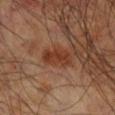Q: Was a biopsy performed?
A: no biopsy performed (imaged during a skin exam)
Q: Who is the patient?
A: male, in their 60s
Q: What is the anatomic site?
A: the right leg
Q: What is the imaging modality?
A: 15 mm crop, total-body photography
Q: What lighting was used for the tile?
A: cross-polarized illumination
Q: How large is the lesion?
A: ~4 mm (longest diameter)